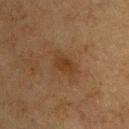Findings:
– workup: total-body-photography surveillance lesion; no biopsy
– patient: male, approximately 75 years of age
– body site: the chest
– acquisition: 15 mm crop, total-body photography
– tile lighting: cross-polarized illumination
– diameter: about 3 mm